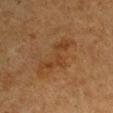notes = imaged on a skin check; not biopsied